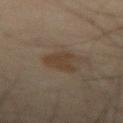The lesion was photographed on a routine skin check and not biopsied; there is no pathology result.